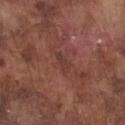{
  "biopsy_status": "not biopsied; imaged during a skin examination",
  "image": {
    "source": "total-body photography crop",
    "field_of_view_mm": 15
  },
  "lesion_size": {
    "long_diameter_mm_approx": 2.5
  },
  "lighting": "white-light",
  "site": "front of the torso",
  "patient": {
    "sex": "male",
    "age_approx": 75
  }
}